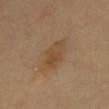{
  "patient": {
    "sex": "male",
    "age_approx": 65
  },
  "lighting": "cross-polarized",
  "lesion_size": {
    "long_diameter_mm_approx": 4.5
  },
  "image": {
    "source": "total-body photography crop",
    "field_of_view_mm": 15
  },
  "site": "chest"
}